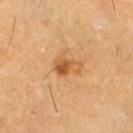notes: total-body-photography surveillance lesion; no biopsy | site: the right thigh | acquisition: 15 mm crop, total-body photography | subject: male, in their 60s.